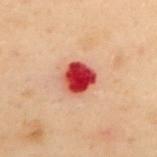Assessment: No biopsy was performed on this lesion — it was imaged during a full skin examination and was not determined to be concerning. Clinical summary: This image is a 15 mm lesion crop taken from a total-body photograph. The subject is a female aged 58–62. The total-body-photography lesion software estimated a shape-asymmetry score of about 0.2 (0 = symmetric). The recorded lesion diameter is about 3.5 mm. On the upper back. This is a cross-polarized tile.